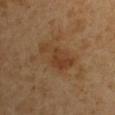Case summary:
* notes: imaged on a skin check; not biopsied
* subject: male, about 50 years old
* site: the right upper arm
* tile lighting: cross-polarized illumination
* diameter: about 5 mm
* image: total-body-photography crop, ~15 mm field of view